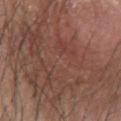Findings:
- follow-up: catalogued during a skin exam; not biopsied
- image source: total-body-photography crop, ~15 mm field of view
- image-analysis metrics: an area of roughly 140 mm² and a symmetry-axis asymmetry near 0.35
- patient: male, aged 53–57
- tile lighting: white-light illumination
- lesion size: about 17.5 mm